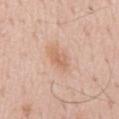Impression:
Part of a total-body skin-imaging series; this lesion was reviewed on a skin check and was not flagged for biopsy.
Clinical summary:
The subject is a male in their mid- to late 50s. Cropped from a whole-body photographic skin survey; the tile spans about 15 mm. This is a white-light tile. Automated tile analysis of the lesion measured a lesion-detection confidence of about 100/100. On the back.